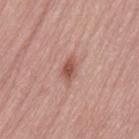Imaged during a routine full-body skin examination; the lesion was not biopsied and no histopathology is available.
The lesion is on the back.
This image is a 15 mm lesion crop taken from a total-body photograph.
A male subject, aged approximately 55.